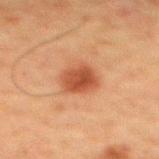Imaged during a routine full-body skin examination; the lesion was not biopsied and no histopathology is available. The lesion is located on the mid back. A 15 mm crop from a total-body photograph taken for skin-cancer surveillance. A male patient aged 58–62.